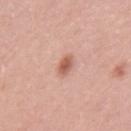The lesion was tiled from a total-body skin photograph and was not biopsied.
Longest diameter approximately 2.5 mm.
This is a white-light tile.
A roughly 15 mm field-of-view crop from a total-body skin photograph.
The lesion-visualizer software estimated a footprint of about 4 mm², an eccentricity of roughly 0.75, and two-axis asymmetry of about 0.25. The software also gave roughly 12 lightness units darker than nearby skin and a normalized border contrast of about 7.5. It also reported a detector confidence of about 100 out of 100 that the crop contains a lesion.
Located on the left upper arm.
A female subject, aged 33 to 37.